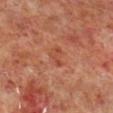Q: What is the imaging modality?
A: total-body-photography crop, ~15 mm field of view
Q: What are the patient's age and sex?
A: male, in their 60s
Q: What did automated image analysis measure?
A: a footprint of about 4 mm²; a lesion color around L≈45 a*≈27 b*≈31 in CIELAB, roughly 6 lightness units darker than nearby skin, and a normalized lesion–skin contrast near 5
Q: Lesion location?
A: the right lower leg
Q: How was the tile lit?
A: cross-polarized illumination
Q: What is the lesion's diameter?
A: ≈2.5 mm